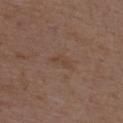Notes:
• biopsy status — imaged on a skin check; not biopsied
• image source — ~15 mm crop, total-body skin-cancer survey
• location — the upper back
• TBP lesion metrics — a mean CIELAB color near L≈42 a*≈17 b*≈26; a border-irregularity index near 4/10, internal color variation of about 0.5 on a 0–10 scale, and peripheral color asymmetry of about 0; an automated nevus-likeness rating near 0 out of 100
• patient — male, roughly 50 years of age
• lesion diameter — ≈3 mm
• illumination — white-light illumination